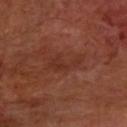follow-up=total-body-photography surveillance lesion; no biopsy | lighting=cross-polarized illumination | image=~15 mm tile from a whole-body skin photo | patient=approximately 65 years of age | lesion size=about 4.5 mm | body site=the left forearm.